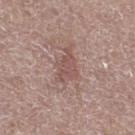Q: Is there a histopathology result?
A: total-body-photography surveillance lesion; no biopsy
Q: Where on the body is the lesion?
A: the right thigh
Q: Lesion size?
A: about 4 mm
Q: Patient demographics?
A: female, aged around 65
Q: What lighting was used for the tile?
A: white-light
Q: What is the imaging modality?
A: 15 mm crop, total-body photography